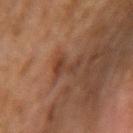<record>
<biopsy_status>not biopsied; imaged during a skin examination</biopsy_status>
<patient>
  <sex>female</sex>
  <age_approx>60</age_approx>
</patient>
<site>right upper arm</site>
<lighting>cross-polarized</lighting>
<automated_metrics>
  <eccentricity>0.9</eccentricity>
  <shape_asymmetry>0.55</shape_asymmetry>
  <border_irregularity_0_10>8.0</border_irregularity_0_10>
  <peripheral_color_asymmetry>0.0</peripheral_color_asymmetry>
  <nevus_likeness_0_100>0</nevus_likeness_0_100>
</automated_metrics>
<image>
  <source>total-body photography crop</source>
  <field_of_view_mm>15</field_of_view_mm>
</image>
<lesion_size>
  <long_diameter_mm_approx>3.5</long_diameter_mm_approx>
</lesion_size>
</record>